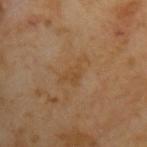The patient is a male approximately 65 years of age. Measured at roughly 4 mm in maximum diameter. A roughly 15 mm field-of-view crop from a total-body skin photograph.